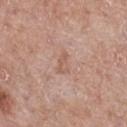workup = total-body-photography surveillance lesion; no biopsy | lighting = white-light illumination | image source = total-body-photography crop, ~15 mm field of view | diameter = about 2.5 mm | patient = male, aged around 70 | automated metrics = a border-irregularity index near 3/10, a within-lesion color-variation index near 0.5/10, and a peripheral color-asymmetry measure near 0; a classifier nevus-likeness of about 0/100 and a detector confidence of about 100 out of 100 that the crop contains a lesion | anatomic site = the chest.